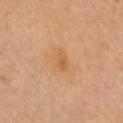workup: catalogued during a skin exam; not biopsied | automated metrics: a lesion area of about 4 mm² and a shape-asymmetry score of about 0.3 (0 = symmetric); an automated nevus-likeness rating near 0 out of 100 | anatomic site: the arm | image source: 15 mm crop, total-body photography | size: about 3 mm | subject: male, in their mid-60s.